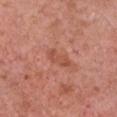Notes:
* workup — total-body-photography surveillance lesion; no biopsy
* imaging modality — ~15 mm crop, total-body skin-cancer survey
* subject — female, approximately 60 years of age
* automated metrics — a peripheral color-asymmetry measure near 0.5
* lighting — white-light illumination
* anatomic site — the chest